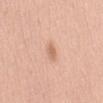| key | value |
|---|---|
| notes | no biopsy performed (imaged during a skin exam) |
| illumination | white-light illumination |
| anatomic site | the abdomen |
| image | 15 mm crop, total-body photography |
| patient | female, roughly 50 years of age |
| size | ~2.5 mm (longest diameter) |